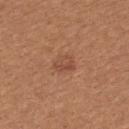notes: total-body-photography surveillance lesion; no biopsy
subject: female, roughly 40 years of age
location: the upper back
lighting: white-light
image: total-body-photography crop, ~15 mm field of view
lesion size: ~3 mm (longest diameter)